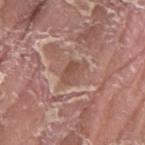Q: Was a biopsy performed?
A: total-body-photography surveillance lesion; no biopsy
Q: Illumination type?
A: white-light
Q: Lesion size?
A: about 3 mm
Q: What are the patient's age and sex?
A: male, aged around 40
Q: What kind of image is this?
A: ~15 mm tile from a whole-body skin photo
Q: What is the anatomic site?
A: the left thigh